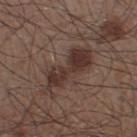biopsy status: imaged on a skin check; not biopsied
diameter: about 7 mm
patient: male, about 60 years old
body site: the left lower leg
image: ~15 mm crop, total-body skin-cancer survey
automated lesion analysis: an average lesion color of about L≈33 a*≈16 b*≈21 (CIELAB) and about 9 CIELAB-L* units darker than the surrounding skin; internal color variation of about 3.5 on a 0–10 scale; a nevus-likeness score of about 40/100 and lesion-presence confidence of about 100/100